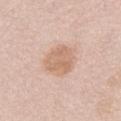The lesion is located on the abdomen.
A female patient approximately 65 years of age.
The recorded lesion diameter is about 4.5 mm.
Imaged with white-light lighting.
A 15 mm close-up extracted from a 3D total-body photography capture.